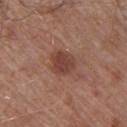Assessment: Part of a total-body skin-imaging series; this lesion was reviewed on a skin check and was not flagged for biopsy. Image and clinical context: Imaged with white-light lighting. This image is a 15 mm lesion crop taken from a total-body photograph. The lesion's longest dimension is about 3.5 mm. The patient is a male aged around 55. From the back.